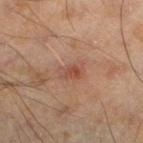A close-up tile cropped from a whole-body skin photograph, about 15 mm across. The tile uses cross-polarized illumination. Located on the left lower leg. Approximately 3 mm at its widest. A male subject aged around 45.